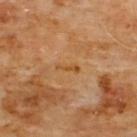notes: no biopsy performed (imaged during a skin exam).